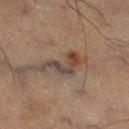A region of skin cropped from a whole-body photographic capture, roughly 15 mm wide. An algorithmic analysis of the crop reported a lesion area of about 9.5 mm² and an outline eccentricity of about 0.9 (0 = round, 1 = elongated). The analysis additionally found a mean CIELAB color near L≈34 a*≈12 b*≈19, roughly 8 lightness units darker than nearby skin, and a lesion-to-skin contrast of about 7.5 (normalized; higher = more distinct). And it measured an automated nevus-likeness rating near 0 out of 100 and lesion-presence confidence of about 50/100. A male patient about 65 years old. About 6.5 mm across. Located on the left thigh.